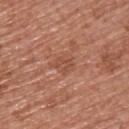Assessment: No biopsy was performed on this lesion — it was imaged during a full skin examination and was not determined to be concerning. Image and clinical context: Cropped from a whole-body photographic skin survey; the tile spans about 15 mm. Located on the back. A male subject in their mid-50s.